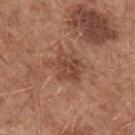  biopsy_status: not biopsied; imaged during a skin examination